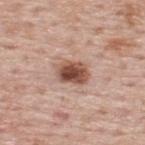biopsy status = imaged on a skin check; not biopsied | image = ~15 mm tile from a whole-body skin photo | patient = male, aged approximately 75 | tile lighting = white-light illumination | location = the back | lesion diameter = about 4 mm.